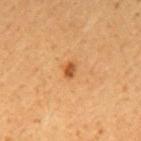Q: Is there a histopathology result?
A: total-body-photography surveillance lesion; no biopsy
Q: Who is the patient?
A: male, roughly 45 years of age
Q: Where on the body is the lesion?
A: the mid back
Q: How large is the lesion?
A: about 2 mm
Q: What is the imaging modality?
A: ~15 mm crop, total-body skin-cancer survey
Q: Automated lesion metrics?
A: a mean CIELAB color near L≈51 a*≈25 b*≈42, a lesion–skin lightness drop of about 12, and a normalized border contrast of about 8.5
Q: How was the tile lit?
A: cross-polarized illumination